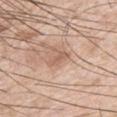Image and clinical context: Cropped from a total-body skin-imaging series; the visible field is about 15 mm. A male subject, about 55 years old. Located on the right upper arm.